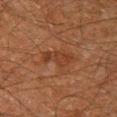No biopsy was performed on this lesion — it was imaged during a full skin examination and was not determined to be concerning.
A region of skin cropped from a whole-body photographic capture, roughly 15 mm wide.
Captured under cross-polarized illumination.
About 4 mm across.
Located on the right lower leg.
The patient is a male aged approximately 60.
The total-body-photography lesion software estimated a footprint of about 5.5 mm², an eccentricity of roughly 0.9, and a shape-asymmetry score of about 0.35 (0 = symmetric). It also reported a normalized lesion–skin contrast near 6. The software also gave border irregularity of about 4 on a 0–10 scale and a within-lesion color-variation index near 2.5/10.